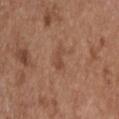notes: imaged on a skin check; not biopsied | image source: 15 mm crop, total-body photography | site: the upper back | subject: male, aged around 55.